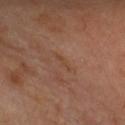Impression: Captured during whole-body skin photography for melanoma surveillance; the lesion was not biopsied. Clinical summary: The lesion is on the head or neck. A female subject aged 68–72. A 15 mm close-up tile from a total-body photography series done for melanoma screening. Captured under cross-polarized illumination.